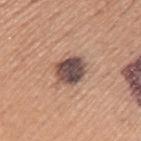notes: imaged on a skin check; not biopsied
tile lighting: white-light illumination
image: ~15 mm crop, total-body skin-cancer survey
patient: male, approximately 45 years of age
lesion size: about 3.5 mm
anatomic site: the left upper arm